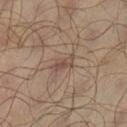notes: imaged on a skin check; not biopsied | location: the leg | diameter: about 3 mm | TBP lesion metrics: a mean CIELAB color near L≈44 a*≈15 b*≈23, roughly 7 lightness units darker than nearby skin, and a normalized lesion–skin contrast near 6; border irregularity of about 3.5 on a 0–10 scale, a color-variation rating of about 1/10, and a peripheral color-asymmetry measure near 0.5 | imaging modality: total-body-photography crop, ~15 mm field of view | illumination: cross-polarized | patient: male, aged around 65.